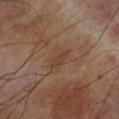Impression:
Captured during whole-body skin photography for melanoma surveillance; the lesion was not biopsied.
Clinical summary:
The lesion-visualizer software estimated a lesion-detection confidence of about 100/100. The subject is a male aged 68–72. On the arm. A 15 mm close-up extracted from a 3D total-body photography capture. Captured under cross-polarized illumination.